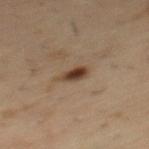Recorded during total-body skin imaging; not selected for excision or biopsy.
A lesion tile, about 15 mm wide, cut from a 3D total-body photograph.
Approximately 3 mm at its widest.
A male patient, aged approximately 55.
The total-body-photography lesion software estimated a footprint of about 4 mm² and a shape-asymmetry score of about 0.25 (0 = symmetric). The analysis additionally found about 13 CIELAB-L* units darker than the surrounding skin and a lesion-to-skin contrast of about 10.5 (normalized; higher = more distinct). The analysis additionally found a lesion-detection confidence of about 100/100.
Imaged with cross-polarized lighting.
From the mid back.